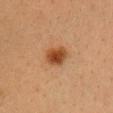{
  "site": "head or neck",
  "image": {
    "source": "total-body photography crop",
    "field_of_view_mm": 15
  },
  "patient": {
    "sex": "female",
    "age_approx": 40
  },
  "lesion_size": {
    "long_diameter_mm_approx": 3.0
  },
  "automated_metrics": {
    "area_mm2_approx": 7.0,
    "shape_asymmetry": 0.2,
    "cielab_L": 41,
    "cielab_a": 22,
    "cielab_b": 34,
    "vs_skin_darker_L": 11.0,
    "vs_skin_contrast_norm": 9.5,
    "color_variation_0_10": 4.5,
    "nevus_likeness_0_100": 100,
    "lesion_detection_confidence_0_100": 100
  }
}